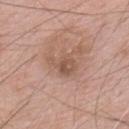Impression:
The lesion was tiled from a total-body skin photograph and was not biopsied.
Clinical summary:
Measured at roughly 4 mm in maximum diameter. Captured under white-light illumination. The subject is a male aged 58–62. A lesion tile, about 15 mm wide, cut from a 3D total-body photograph. The lesion is on the mid back. An algorithmic analysis of the crop reported an outline eccentricity of about 0.8 (0 = round, 1 = elongated) and a shape-asymmetry score of about 0.4 (0 = symmetric). The analysis additionally found an automated nevus-likeness rating near 0 out of 100 and lesion-presence confidence of about 100/100.